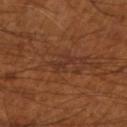workup: total-body-photography surveillance lesion; no biopsy | location: the right forearm | subject: male, aged approximately 55 | size: about 3 mm | illumination: cross-polarized | imaging modality: ~15 mm crop, total-body skin-cancer survey | image-analysis metrics: a lesion area of about 3 mm² and an eccentricity of roughly 0.85; a lesion color around L≈31 a*≈21 b*≈27 in CIELAB.